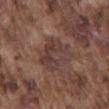Assessment:
Part of a total-body skin-imaging series; this lesion was reviewed on a skin check and was not flagged for biopsy.
Clinical summary:
From the mid back. Automated image analysis of the tile measured a mean CIELAB color near L≈39 a*≈18 b*≈21 and a normalized lesion–skin contrast near 7. Approximately 5 mm at its widest. A male patient, aged approximately 75. A 15 mm crop from a total-body photograph taken for skin-cancer surveillance. Captured under white-light illumination.